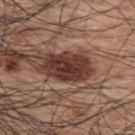Captured during whole-body skin photography for melanoma surveillance; the lesion was not biopsied.
The total-body-photography lesion software estimated a border-irregularity rating of about 2.5/10 and a peripheral color-asymmetry measure near 1.5.
The lesion is located on the back.
Imaged with white-light lighting.
The subject is a male aged approximately 70.
A roughly 15 mm field-of-view crop from a total-body skin photograph.
Measured at roughly 5.5 mm in maximum diameter.